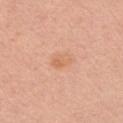Captured during whole-body skin photography for melanoma surveillance; the lesion was not biopsied. Cropped from a total-body skin-imaging series; the visible field is about 15 mm. A male patient, about 30 years old. Captured under white-light illumination. Located on the left upper arm.